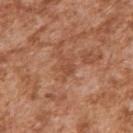| field | value |
|---|---|
| tile lighting | white-light |
| lesion size | ~2.5 mm (longest diameter) |
| patient | male, in their mid- to late 40s |
| image source | ~15 mm tile from a whole-body skin photo |
| location | the right upper arm |